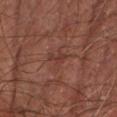biopsy_status: not biopsied; imaged during a skin examination
lesion_size:
  long_diameter_mm_approx: 2.5
image:
  source: total-body photography crop
  field_of_view_mm: 15
lighting: cross-polarized
patient:
  sex: male
  age_approx: 65
site: left forearm